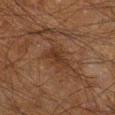{"biopsy_status": "not biopsied; imaged during a skin examination", "lesion_size": {"long_diameter_mm_approx": 3.5}, "image": {"source": "total-body photography crop", "field_of_view_mm": 15}, "lighting": "cross-polarized", "site": "right lower leg", "patient": {"sex": "male", "age_approx": 60}}